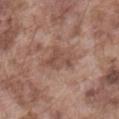follow-up=imaged on a skin check; not biopsied | illumination=white-light illumination | subject=male, approximately 75 years of age | diameter=about 4 mm | anatomic site=the front of the torso | imaging modality=15 mm crop, total-body photography.